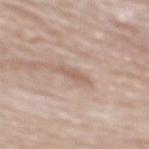The lesion's longest dimension is about 2.5 mm. This is a white-light tile. The lesion is located on the mid back. Cropped from a whole-body photographic skin survey; the tile spans about 15 mm. A male subject, about 80 years old.